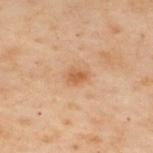Clinical summary:
Cropped from a total-body skin-imaging series; the visible field is about 15 mm. A male subject, aged 48 to 52. Located on the upper back.